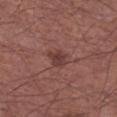Impression:
Imaged during a routine full-body skin examination; the lesion was not biopsied and no histopathology is available.
Context:
The recorded lesion diameter is about 3 mm. Automated tile analysis of the lesion measured an area of roughly 4 mm², an eccentricity of roughly 0.7, and two-axis asymmetry of about 0.3. And it measured an average lesion color of about L≈38 a*≈22 b*≈21 (CIELAB), about 9 CIELAB-L* units darker than the surrounding skin, and a normalized border contrast of about 7.5. And it measured a nevus-likeness score of about 65/100 and a detector confidence of about 100 out of 100 that the crop contains a lesion. A 15 mm close-up extracted from a 3D total-body photography capture. From the leg. Imaged with white-light lighting. The patient is a male in their mid- to late 60s.